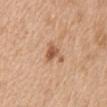  biopsy_status: not biopsied; imaged during a skin examination
  automated_metrics:
    area_mm2_approx: 5.0
    eccentricity: 0.8
    shape_asymmetry: 0.5
  lesion_size:
    long_diameter_mm_approx: 4.0
  lighting: white-light
  patient:
    sex: female
    age_approx: 55
  image:
    source: total-body photography crop
    field_of_view_mm: 15
  site: front of the torso